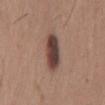| key | value |
|---|---|
| notes | total-body-photography surveillance lesion; no biopsy |
| TBP lesion metrics | an area of roughly 10 mm² and an eccentricity of roughly 0.9; an average lesion color of about L≈42 a*≈18 b*≈22 (CIELAB), a lesion–skin lightness drop of about 15, and a lesion-to-skin contrast of about 12 (normalized; higher = more distinct); border irregularity of about 2.5 on a 0–10 scale, internal color variation of about 6.5 on a 0–10 scale, and radial color variation of about 2; an automated nevus-likeness rating near 75 out of 100 and a detector confidence of about 100 out of 100 that the crop contains a lesion |
| body site | the back |
| patient | male, in their mid-60s |
| imaging modality | 15 mm crop, total-body photography |